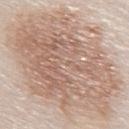Captured during whole-body skin photography for melanoma surveillance; the lesion was not biopsied.
The recorded lesion diameter is about 15.5 mm.
A male subject, aged 78 to 82.
A lesion tile, about 15 mm wide, cut from a 3D total-body photograph.
From the abdomen.
The tile uses white-light illumination.